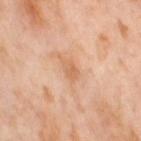Part of a total-body skin-imaging series; this lesion was reviewed on a skin check and was not flagged for biopsy. The tile uses cross-polarized illumination. The subject is a female about 55 years old. Automated image analysis of the tile measured a shape eccentricity near 0.8 and two-axis asymmetry of about 0.2. The analysis additionally found border irregularity of about 2 on a 0–10 scale and a peripheral color-asymmetry measure near 0.5. It also reported a classifier nevus-likeness of about 0/100. The lesion's longest dimension is about 2.5 mm. The lesion is on the right thigh. A 15 mm close-up extracted from a 3D total-body photography capture.